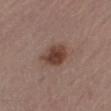- site · the leg
- image source · total-body-photography crop, ~15 mm field of view
- subject · male, in their mid- to late 60s